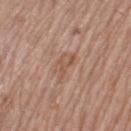{"biopsy_status": "not biopsied; imaged during a skin examination", "lesion_size": {"long_diameter_mm_approx": 3.5}, "lighting": "white-light", "image": {"source": "total-body photography crop", "field_of_view_mm": 15}, "patient": {"sex": "female", "age_approx": 60}, "site": "left thigh"}